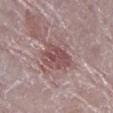Impression:
The lesion was photographed on a routine skin check and not biopsied; there is no pathology result.
Context:
The subject is a female in their mid-60s. The lesion-visualizer software estimated an outline eccentricity of about 0.75 (0 = round, 1 = elongated) and a shape-asymmetry score of about 0.25 (0 = symmetric). The analysis additionally found a lesion color around L≈50 a*≈21 b*≈18 in CIELAB and about 10 CIELAB-L* units darker than the surrounding skin. The analysis additionally found a border-irregularity index near 3/10 and radial color variation of about 1.5. The analysis additionally found a classifier nevus-likeness of about 5/100 and a lesion-detection confidence of about 95/100. Cropped from a whole-body photographic skin survey; the tile spans about 15 mm. About 5 mm across. Captured under white-light illumination. From the left lower leg.